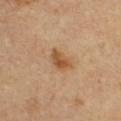<tbp_lesion>
<biopsy_status>not biopsied; imaged during a skin examination</biopsy_status>
<image>
  <source>total-body photography crop</source>
  <field_of_view_mm>15</field_of_view_mm>
</image>
<lesion_size>
  <long_diameter_mm_approx>3.5</long_diameter_mm_approx>
</lesion_size>
<automated_metrics>
  <cielab_L>51</cielab_L>
  <cielab_a>19</cielab_a>
  <cielab_b>36</cielab_b>
  <vs_skin_darker_L>10.0</vs_skin_darker_L>
  <vs_skin_contrast_norm>8.0</vs_skin_contrast_norm>
  <border_irregularity_0_10>2.5</border_irregularity_0_10>
  <color_variation_0_10>3.0</color_variation_0_10>
  <peripheral_color_asymmetry>1.0</peripheral_color_asymmetry>
  <nevus_likeness_0_100>80</nevus_likeness_0_100>
  <lesion_detection_confidence_0_100>100</lesion_detection_confidence_0_100>
</automated_metrics>
<site>chest</site>
<lighting>cross-polarized</lighting>
<patient>
  <sex>male</sex>
  <age_approx>55</age_approx>
</patient>
</tbp_lesion>